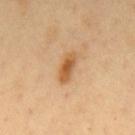The lesion was photographed on a routine skin check and not biopsied; there is no pathology result. This image is a 15 mm lesion crop taken from a total-body photograph. Imaged with cross-polarized lighting. A male subject, aged 48–52. Located on the mid back.